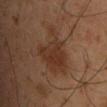Impression: Recorded during total-body skin imaging; not selected for excision or biopsy. Acquisition and patient details: The lesion is on the back. A male patient approximately 50 years of age. This image is a 15 mm lesion crop taken from a total-body photograph. Captured under cross-polarized illumination. About 5.5 mm across. An algorithmic analysis of the crop reported an eccentricity of roughly 0.45 and a shape-asymmetry score of about 0.45 (0 = symmetric). The software also gave about 7 CIELAB-L* units darker than the surrounding skin. It also reported a border-irregularity index near 6.5/10 and radial color variation of about 1. The software also gave a lesion-detection confidence of about 100/100.